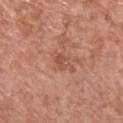Captured under white-light illumination. The lesion is located on the chest. A male patient, aged 68 to 72. This image is a 15 mm lesion crop taken from a total-body photograph.